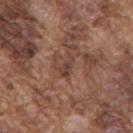Case summary:
- notes: imaged on a skin check; not biopsied
- lighting: white-light illumination
- site: the back
- subject: male, roughly 75 years of age
- automated metrics: an average lesion color of about L≈41 a*≈19 b*≈25 (CIELAB), a lesion–skin lightness drop of about 8, and a lesion-to-skin contrast of about 7 (normalized; higher = more distinct)
- image: ~15 mm crop, total-body skin-cancer survey
- size: ~3 mm (longest diameter)